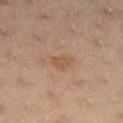Case summary:
• notes — no biopsy performed (imaged during a skin exam)
• image — total-body-photography crop, ~15 mm field of view
• patient — female, aged around 20
• site — the right lower leg